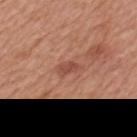follow-up=no biopsy performed (imaged during a skin exam).